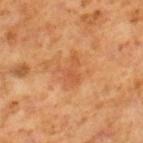follow-up = total-body-photography surveillance lesion; no biopsy | location = the right lower leg | image source = total-body-photography crop, ~15 mm field of view | patient = male, aged around 60 | tile lighting = cross-polarized illumination | lesion diameter = ≈3.5 mm.